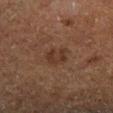workup: catalogued during a skin exam; not biopsied
patient: male, aged approximately 75
anatomic site: the left lower leg
image-analysis metrics: a footprint of about 6 mm² and an eccentricity of roughly 0.2
size: ≈2.5 mm
tile lighting: cross-polarized illumination
image source: ~15 mm tile from a whole-body skin photo